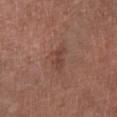| key | value |
|---|---|
| follow-up | imaged on a skin check; not biopsied |
| patient | male, about 70 years old |
| image-analysis metrics | an area of roughly 5 mm², an eccentricity of roughly 0.8, and a symmetry-axis asymmetry near 0.35; a lesion color around L≈43 a*≈21 b*≈25 in CIELAB, a lesion–skin lightness drop of about 7, and a normalized border contrast of about 5.5 |
| imaging modality | ~15 mm crop, total-body skin-cancer survey |
| diameter | ≈3.5 mm |
| location | the left lower leg |
| lighting | white-light illumination |